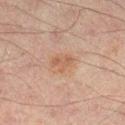follow-up: imaged on a skin check; not biopsied | imaging modality: ~15 mm tile from a whole-body skin photo | patient: male, in their 30s | body site: the right lower leg.